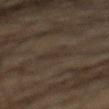The lesion was photographed on a routine skin check and not biopsied; there is no pathology result. A close-up tile cropped from a whole-body skin photograph, about 15 mm across. A male patient, approximately 65 years of age. The lesion is on the abdomen. Longest diameter approximately 3 mm. Imaged with cross-polarized lighting.